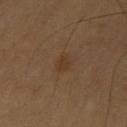Impression: This lesion was catalogued during total-body skin photography and was not selected for biopsy. Image and clinical context: A 15 mm crop from a total-body photograph taken for skin-cancer surveillance. The lesion's longest dimension is about 2.5 mm. The subject is a female in their 70s. The lesion is on the leg. Imaged with cross-polarized lighting.